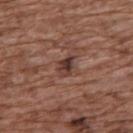The lesion was tiled from a total-body skin photograph and was not biopsied. A female subject, aged 73 to 77. A roughly 15 mm field-of-view crop from a total-body skin photograph. On the upper back.